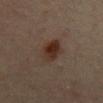Q: What is the anatomic site?
A: the front of the torso
Q: Who is the patient?
A: female, about 65 years old
Q: Automated lesion metrics?
A: an area of roughly 8 mm², a shape eccentricity near 0.8, and a symmetry-axis asymmetry near 0.15; border irregularity of about 2 on a 0–10 scale, a within-lesion color-variation index near 4.5/10, and peripheral color asymmetry of about 1.5
Q: What is the lesion's diameter?
A: about 4 mm
Q: What lighting was used for the tile?
A: cross-polarized illumination
Q: What kind of image is this?
A: ~15 mm tile from a whole-body skin photo